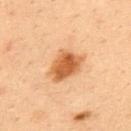Imaged during a routine full-body skin examination; the lesion was not biopsied and no histopathology is available. A male subject in their mid- to late 30s. A roughly 15 mm field-of-view crop from a total-body skin photograph. Located on the back.